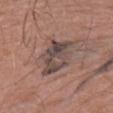Clinical impression: Recorded during total-body skin imaging; not selected for excision or biopsy. Image and clinical context: A roughly 15 mm field-of-view crop from a total-body skin photograph. An algorithmic analysis of the crop reported two-axis asymmetry of about 0.3. The software also gave a mean CIELAB color near L≈45 a*≈14 b*≈19, roughly 11 lightness units darker than nearby skin, and a normalized border contrast of about 9. The analysis additionally found a color-variation rating of about 7/10 and radial color variation of about 2.5. The analysis additionally found an automated nevus-likeness rating near 0 out of 100 and a lesion-detection confidence of about 80/100. Captured under white-light illumination. About 6 mm across. On the right upper arm. A male patient, aged 68 to 72.